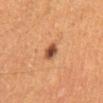biopsy status = no biopsy performed (imaged during a skin exam)
lighting = cross-polarized
anatomic site = the right lower leg
subject = male, aged 63 to 67
lesion diameter = about 2 mm
automated metrics = a footprint of about 4 mm², a shape eccentricity near 0.4, and a symmetry-axis asymmetry near 0.25; a lesion color around L≈44 a*≈23 b*≈32 in CIELAB, about 14 CIELAB-L* units darker than the surrounding skin, and a normalized border contrast of about 10.5; border irregularity of about 2 on a 0–10 scale, internal color variation of about 6.5 on a 0–10 scale, and radial color variation of about 2
acquisition = 15 mm crop, total-body photography